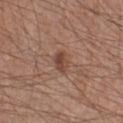Notes:
• notes: imaged on a skin check; not biopsied
• patient: male, in their mid- to late 50s
• location: the leg
• acquisition: 15 mm crop, total-body photography